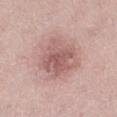Clinical impression: Imaged during a routine full-body skin examination; the lesion was not biopsied and no histopathology is available. Clinical summary: The patient is a female aged approximately 40. A 15 mm crop from a total-body photograph taken for skin-cancer surveillance. On the right thigh.